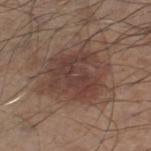Q: Is there a histopathology result?
A: imaged on a skin check; not biopsied
Q: What are the patient's age and sex?
A: male, aged 58 to 62
Q: Lesion location?
A: the right thigh
Q: What kind of image is this?
A: total-body-photography crop, ~15 mm field of view